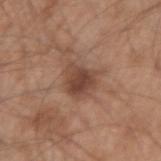The lesion was tiled from a total-body skin photograph and was not biopsied.
The lesion is located on the right upper arm.
A region of skin cropped from a whole-body photographic capture, roughly 15 mm wide.
The subject is a male approximately 55 years of age.
Automated tile analysis of the lesion measured an area of roughly 9.5 mm², an eccentricity of roughly 0.35, and a shape-asymmetry score of about 0.3 (0 = symmetric). And it measured a classifier nevus-likeness of about 55/100 and a lesion-detection confidence of about 100/100.